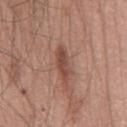Part of a total-body skin-imaging series; this lesion was reviewed on a skin check and was not flagged for biopsy.
A lesion tile, about 15 mm wide, cut from a 3D total-body photograph.
The lesion is located on the chest.
A male patient, aged 53 to 57.